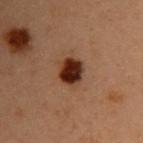Part of a total-body skin-imaging series; this lesion was reviewed on a skin check and was not flagged for biopsy. Captured under cross-polarized illumination. The patient is a female aged approximately 40. The recorded lesion diameter is about 3 mm. Located on the upper back. A lesion tile, about 15 mm wide, cut from a 3D total-body photograph.